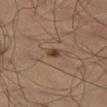lesion size — about 2 mm
acquisition — total-body-photography crop, ~15 mm field of view
tile lighting — cross-polarized
location — the leg
automated metrics — a border-irregularity index near 2/10, internal color variation of about 1 on a 0–10 scale, and a peripheral color-asymmetry measure near 0.5; a nevus-likeness score of about 95/100 and a detector confidence of about 100 out of 100 that the crop contains a lesion
patient — male, aged 63 to 67